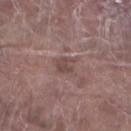Impression: Captured during whole-body skin photography for melanoma surveillance; the lesion was not biopsied.